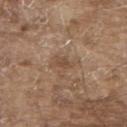Assessment:
The lesion was photographed on a routine skin check and not biopsied; there is no pathology result.
Acquisition and patient details:
Approximately 2.5 mm at its widest. An algorithmic analysis of the crop reported a mean CIELAB color near L≈48 a*≈17 b*≈29, about 7 CIELAB-L* units darker than the surrounding skin, and a normalized lesion–skin contrast near 6. And it measured a color-variation rating of about 0.5/10. The analysis additionally found an automated nevus-likeness rating near 0 out of 100 and a lesion-detection confidence of about 95/100. From the upper back. The subject is a male approximately 80 years of age. Cropped from a whole-body photographic skin survey; the tile spans about 15 mm.